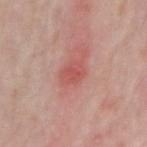The lesion was photographed on a routine skin check and not biopsied; there is no pathology result. Cropped from a total-body skin-imaging series; the visible field is about 15 mm. Located on the right forearm. A male subject aged around 75.